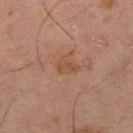The lesion was photographed on a routine skin check and not biopsied; there is no pathology result. Automated image analysis of the tile measured an average lesion color of about L≈39 a*≈18 b*≈26 (CIELAB), roughly 5 lightness units darker than nearby skin, and a normalized lesion–skin contrast near 5.5. It also reported a nevus-likeness score of about 0/100 and a lesion-detection confidence of about 100/100. On the leg. A close-up tile cropped from a whole-body skin photograph, about 15 mm across. Measured at roughly 2.5 mm in maximum diameter. The tile uses cross-polarized illumination. A male patient, about 65 years old.